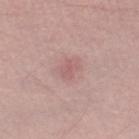Clinical impression: This lesion was catalogued during total-body skin photography and was not selected for biopsy. Context: The patient is a male aged 38–42. A close-up tile cropped from a whole-body skin photograph, about 15 mm across. Captured under white-light illumination. Automated tile analysis of the lesion measured an area of roughly 5 mm², a shape eccentricity near 0.55, and a shape-asymmetry score of about 0.25 (0 = symmetric). Located on the right thigh.